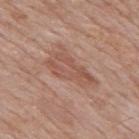Assessment: Imaged during a routine full-body skin examination; the lesion was not biopsied and no histopathology is available.